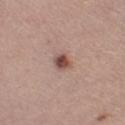Imaged during a routine full-body skin examination; the lesion was not biopsied and no histopathology is available. Longest diameter approximately 2.5 mm. Located on the left thigh. A female subject about 45 years old. The tile uses white-light illumination. A 15 mm crop from a total-body photograph taken for skin-cancer surveillance.